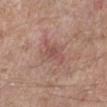- tile lighting — white-light
- automated metrics — a lesion color around L≈51 a*≈22 b*≈25 in CIELAB, roughly 7 lightness units darker than nearby skin, and a normalized border contrast of about 5; a classifier nevus-likeness of about 0/100 and a lesion-detection confidence of about 100/100
- imaging modality — 15 mm crop, total-body photography
- patient — male, approximately 55 years of age
- anatomic site — the left lower leg